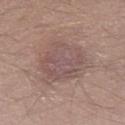The lesion was photographed on a routine skin check and not biopsied; there is no pathology result. Automated tile analysis of the lesion measured a lesion area of about 26 mm² and a shape eccentricity near 0.45. It also reported a mean CIELAB color near L≈52 a*≈17 b*≈20, roughly 7 lightness units darker than nearby skin, and a normalized lesion–skin contrast near 5.5. It also reported a detector confidence of about 100 out of 100 that the crop contains a lesion. Located on the right thigh. A male patient aged approximately 40. The tile uses white-light illumination. A region of skin cropped from a whole-body photographic capture, roughly 15 mm wide. Longest diameter approximately 6.5 mm.